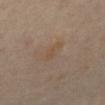{
  "biopsy_status": "not biopsied; imaged during a skin examination",
  "lesion_size": {
    "long_diameter_mm_approx": 3.0
  },
  "patient": {
    "sex": "male",
    "age_approx": 65
  },
  "image": {
    "source": "total-body photography crop",
    "field_of_view_mm": 15
  },
  "automated_metrics": {
    "eccentricity": 0.9,
    "shape_asymmetry": 0.35,
    "border_irregularity_0_10": 4.5,
    "color_variation_0_10": 0.5,
    "peripheral_color_asymmetry": 0.0,
    "nevus_likeness_0_100": 0,
    "lesion_detection_confidence_0_100": 100
  },
  "lighting": "cross-polarized",
  "site": "front of the torso"
}